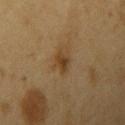Impression:
No biopsy was performed on this lesion — it was imaged during a full skin examination and was not determined to be concerning.
Clinical summary:
The lesion is on the right upper arm. A 15 mm close-up extracted from a 3D total-body photography capture. Measured at roughly 2.5 mm in maximum diameter. The subject is a male roughly 60 years of age. Automated image analysis of the tile measured a lesion area of about 3.5 mm². The analysis additionally found a border-irregularity rating of about 3/10, a within-lesion color-variation index near 2.5/10, and a peripheral color-asymmetry measure near 1. The software also gave a classifier nevus-likeness of about 50/100. The tile uses cross-polarized illumination.